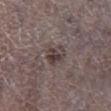Clinical impression: No biopsy was performed on this lesion — it was imaged during a full skin examination and was not determined to be concerning. Acquisition and patient details: This image is a 15 mm lesion crop taken from a total-body photograph. The tile uses white-light illumination. The lesion is located on the left lower leg. A male patient aged 68 to 72.